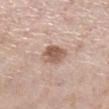<lesion>
<biopsy_status>not biopsied; imaged during a skin examination</biopsy_status>
<automated_metrics>
  <area_mm2_approx>7.0</area_mm2_approx>
  <shape_asymmetry>0.15</shape_asymmetry>
  <cielab_L>57</cielab_L>
  <cielab_a>19</cielab_a>
  <cielab_b>27</cielab_b>
  <vs_skin_darker_L>13.0</vs_skin_darker_L>
  <vs_skin_contrast_norm>8.5</vs_skin_contrast_norm>
  <nevus_likeness_0_100>85</nevus_likeness_0_100>
  <lesion_detection_confidence_0_100>100</lesion_detection_confidence_0_100>
</automated_metrics>
<site>right lower leg</site>
<lighting>white-light</lighting>
<patient>
  <sex>female</sex>
  <age_approx>65</age_approx>
</patient>
<image>
  <source>total-body photography crop</source>
  <field_of_view_mm>15</field_of_view_mm>
</image>
</lesion>